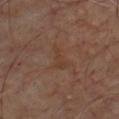Located on the chest.
Automated tile analysis of the lesion measured a nevus-likeness score of about 0/100 and a lesion-detection confidence of about 100/100.
A roughly 15 mm field-of-view crop from a total-body skin photograph.
A male patient about 70 years old.
The lesion's longest dimension is about 3 mm.
This is a cross-polarized tile.